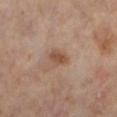No biopsy was performed on this lesion — it was imaged during a full skin examination and was not determined to be concerning.